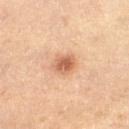Clinical impression:
The lesion was tiled from a total-body skin photograph and was not biopsied.
Context:
The recorded lesion diameter is about 3 mm. The lesion is on the right thigh. A female subject aged 43–47. A roughly 15 mm field-of-view crop from a total-body skin photograph. An algorithmic analysis of the crop reported a lesion area of about 5.5 mm², an outline eccentricity of about 0.45 (0 = round, 1 = elongated), and a shape-asymmetry score of about 0.15 (0 = symmetric). The software also gave roughly 13 lightness units darker than nearby skin and a normalized lesion–skin contrast near 8. Imaged with cross-polarized lighting.